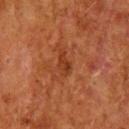A 15 mm close-up extracted from a 3D total-body photography capture. The lesion's longest dimension is about 3 mm. This is a cross-polarized tile. From the head or neck. The patient is a male aged 63 to 67. An algorithmic analysis of the crop reported about 6 CIELAB-L* units darker than the surrounding skin and a normalized lesion–skin contrast near 6.5. It also reported a classifier nevus-likeness of about 0/100.